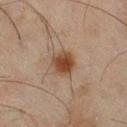{
  "biopsy_status": "not biopsied; imaged during a skin examination",
  "lighting": "cross-polarized",
  "site": "right thigh",
  "automated_metrics": {
    "area_mm2_approx": 7.5,
    "shape_asymmetry": 0.15,
    "border_irregularity_0_10": 1.5,
    "color_variation_0_10": 3.5,
    "peripheral_color_asymmetry": 1.0,
    "lesion_detection_confidence_0_100": 100
  },
  "lesion_size": {
    "long_diameter_mm_approx": 3.5
  },
  "patient": {
    "sex": "male",
    "age_approx": 45
  },
  "image": {
    "source": "total-body photography crop",
    "field_of_view_mm": 15
  }
}